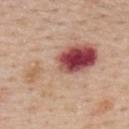Case summary:
• subject · male, approximately 60 years of age
• site · the upper back
• imaging modality · ~15 mm crop, total-body skin-cancer survey